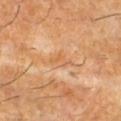follow-up=no biopsy performed (imaged during a skin exam)
size=≈3 mm
anatomic site=the right lower leg
patient=male, aged 58 to 62
imaging modality=total-body-photography crop, ~15 mm field of view